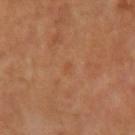• biopsy status: catalogued during a skin exam; not biopsied
• patient: female, aged around 60
• anatomic site: the left arm
• size: ≈1 mm
• automated lesion analysis: a lesion area of about 1 mm², a shape eccentricity near 0.75, and two-axis asymmetry of about 0.5; a classifier nevus-likeness of about 0/100 and a detector confidence of about 100 out of 100 that the crop contains a lesion
• illumination: cross-polarized
• acquisition: 15 mm crop, total-body photography